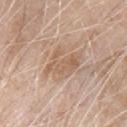Findings:
• workup: total-body-photography surveillance lesion; no biopsy
• site: the chest
• imaging modality: ~15 mm crop, total-body skin-cancer survey
• size: ≈4.5 mm
• subject: male, in their 80s